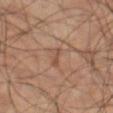The lesion was tiled from a total-body skin photograph and was not biopsied. Cropped from a whole-body photographic skin survey; the tile spans about 15 mm. A male patient, aged approximately 60. The lesion is on the left thigh.